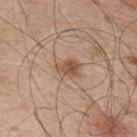This lesion was catalogued during total-body skin photography and was not selected for biopsy. A 15 mm close-up extracted from a 3D total-body photography capture. The tile uses white-light illumination. A male patient, aged 48–52. The lesion is on the upper back. Approximately 3 mm at its widest.